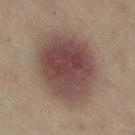Imaged during a routine full-body skin examination; the lesion was not biopsied and no histopathology is available. Automated image analysis of the tile measured a mean CIELAB color near L≈38 a*≈15 b*≈18, roughly 11 lightness units darker than nearby skin, and a lesion-to-skin contrast of about 10 (normalized; higher = more distinct). Approximately 9 mm at its widest. On the right lower leg. A 15 mm close-up tile from a total-body photography series done for melanoma screening. The tile uses cross-polarized illumination. The subject is a female aged 43–47.